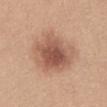Part of a total-body skin-imaging series; this lesion was reviewed on a skin check and was not flagged for biopsy.
About 6 mm across.
The lesion-visualizer software estimated an average lesion color of about L≈56 a*≈22 b*≈29 (CIELAB), about 12 CIELAB-L* units darker than the surrounding skin, and a lesion-to-skin contrast of about 8 (normalized; higher = more distinct). The analysis additionally found border irregularity of about 2 on a 0–10 scale and a color-variation rating of about 5.5/10. It also reported a classifier nevus-likeness of about 90/100 and lesion-presence confidence of about 100/100.
A close-up tile cropped from a whole-body skin photograph, about 15 mm across.
The tile uses white-light illumination.
The lesion is on the abdomen.
A female subject, roughly 30 years of age.